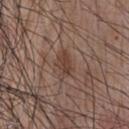This lesion was catalogued during total-body skin photography and was not selected for biopsy.
The lesion is on the chest.
A 15 mm close-up extracted from a 3D total-body photography capture.
The subject is a male about 55 years old.